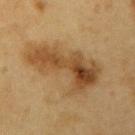* follow-up: imaged on a skin check; not biopsied
* image source: ~15 mm crop, total-body skin-cancer survey
* patient: male, in their 60s
* illumination: cross-polarized
* diameter: ≈8.5 mm
* body site: the arm